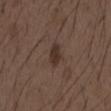Clinical impression:
Recorded during total-body skin imaging; not selected for excision or biopsy.
Context:
From the chest. A lesion tile, about 15 mm wide, cut from a 3D total-body photograph. A male subject, about 50 years old. The lesion-visualizer software estimated a lesion area of about 4.5 mm², an outline eccentricity of about 0.85 (0 = round, 1 = elongated), and a shape-asymmetry score of about 0.3 (0 = symmetric). It also reported a mean CIELAB color near L≈32 a*≈15 b*≈21, about 9 CIELAB-L* units darker than the surrounding skin, and a lesion-to-skin contrast of about 8.5 (normalized; higher = more distinct). The software also gave a nevus-likeness score of about 80/100. Captured under white-light illumination.